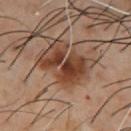The lesion is located on the chest.
A male subject in their mid- to late 50s.
Approximately 5.5 mm at its widest.
The lesion-visualizer software estimated an area of roughly 13 mm², a shape eccentricity near 0.75, and a symmetry-axis asymmetry near 0.45. It also reported a lesion color around L≈38 a*≈21 b*≈29 in CIELAB, about 13 CIELAB-L* units darker than the surrounding skin, and a lesion-to-skin contrast of about 10.5 (normalized; higher = more distinct). It also reported a color-variation rating of about 5/10 and peripheral color asymmetry of about 1.5.
A lesion tile, about 15 mm wide, cut from a 3D total-body photograph.
The tile uses cross-polarized illumination.